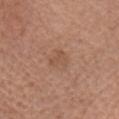Findings:
• workup · imaged on a skin check; not biopsied
• image source · 15 mm crop, total-body photography
• lighting · white-light
• subject · female, in their mid- to late 70s
• anatomic site · the chest